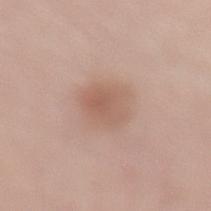Clinical impression:
The lesion was tiled from a total-body skin photograph and was not biopsied.
Image and clinical context:
A male subject aged 68–72. Cropped from a whole-body photographic skin survey; the tile spans about 15 mm. The lesion is located on the back. An algorithmic analysis of the crop reported an outline eccentricity of about 0.5 (0 = round, 1 = elongated) and a symmetry-axis asymmetry near 0.15. And it measured an automated nevus-likeness rating near 80 out of 100 and a lesion-detection confidence of about 100/100. Imaged with white-light lighting. The lesion's longest dimension is about 3.5 mm.